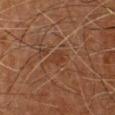Clinical impression: Part of a total-body skin-imaging series; this lesion was reviewed on a skin check and was not flagged for biopsy.